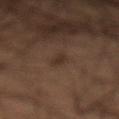* tile lighting: cross-polarized illumination
* image source: total-body-photography crop, ~15 mm field of view
* patient: male, aged approximately 55
* lesion size: about 2.5 mm
* TBP lesion metrics: a border-irregularity index near 5/10, a color-variation rating of about 0/10, and a peripheral color-asymmetry measure near 0; lesion-presence confidence of about 95/100
* anatomic site: the abdomen